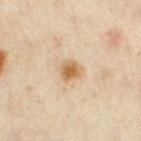This lesion was catalogued during total-body skin photography and was not selected for biopsy. The recorded lesion diameter is about 2.5 mm. The lesion is on the right thigh. A female subject, about 35 years old. A roughly 15 mm field-of-view crop from a total-body skin photograph. This is a cross-polarized tile.